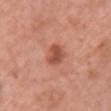Captured under white-light illumination.
From the chest.
Automated tile analysis of the lesion measured a border-irregularity index near 2/10, a within-lesion color-variation index near 3.5/10, and peripheral color asymmetry of about 1.5.
Cropped from a whole-body photographic skin survey; the tile spans about 15 mm.
A female patient, aged around 60.